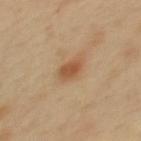Imaged during a routine full-body skin examination; the lesion was not biopsied and no histopathology is available. A female patient, aged around 40. Longest diameter approximately 3 mm. The lesion is located on the upper back. Automated image analysis of the tile measured about 10 CIELAB-L* units darker than the surrounding skin and a lesion-to-skin contrast of about 7.5 (normalized; higher = more distinct). A 15 mm crop from a total-body photograph taken for skin-cancer surveillance. Captured under cross-polarized illumination.